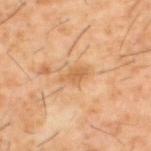follow-up=catalogued during a skin exam; not biopsied | lighting=cross-polarized | anatomic site=the upper back | lesion size=about 3 mm | image source=15 mm crop, total-body photography | subject=male, roughly 60 years of age.